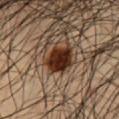{
  "biopsy_status": "not biopsied; imaged during a skin examination",
  "image": {
    "source": "total-body photography crop",
    "field_of_view_mm": 15
  },
  "lighting": "cross-polarized",
  "patient": {
    "sex": "male",
    "age_approx": 50
  },
  "site": "abdomen",
  "lesion_size": {
    "long_diameter_mm_approx": 3.5
  }
}